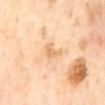Impression:
The lesion was tiled from a total-body skin photograph and was not biopsied.
Clinical summary:
Measured at roughly 2.5 mm in maximum diameter. The patient is a female in their mid-50s. The lesion is located on the mid back. A close-up tile cropped from a whole-body skin photograph, about 15 mm across. Imaged with cross-polarized lighting.